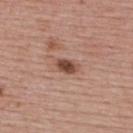Assessment: Part of a total-body skin-imaging series; this lesion was reviewed on a skin check and was not flagged for biopsy. Acquisition and patient details: The lesion is located on the upper back. A close-up tile cropped from a whole-body skin photograph, about 15 mm across. The lesion's longest dimension is about 3 mm. This is a white-light tile. The patient is a female aged 53–57.